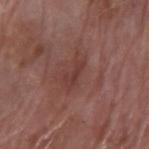Assessment: Captured during whole-body skin photography for melanoma surveillance; the lesion was not biopsied.